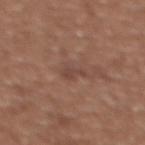biopsy status: imaged on a skin check; not biopsied | patient: female, aged approximately 35 | lesion diameter: ≈3 mm | image: ~15 mm tile from a whole-body skin photo | location: the chest.